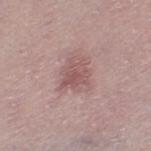biopsy status: total-body-photography surveillance lesion; no biopsy | patient: female, roughly 40 years of age | illumination: white-light | acquisition: ~15 mm tile from a whole-body skin photo | location: the left thigh | lesion size: ≈4 mm.